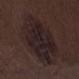Recorded during total-body skin imaging; not selected for excision or biopsy.
A 15 mm crop from a total-body photograph taken for skin-cancer surveillance.
A male subject, aged approximately 70.
From the left thigh.